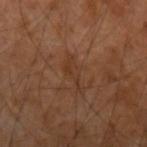Recorded during total-body skin imaging; not selected for excision or biopsy. A male subject, aged 53–57. Imaged with cross-polarized lighting. The lesion's longest dimension is about 4 mm. This image is a 15 mm lesion crop taken from a total-body photograph. The lesion is on the left forearm.